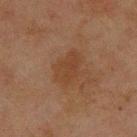Captured during whole-body skin photography for melanoma surveillance; the lesion was not biopsied.
The tile uses cross-polarized illumination.
A close-up tile cropped from a whole-body skin photograph, about 15 mm across.
The lesion's longest dimension is about 4 mm.
Automated image analysis of the tile measured a border-irregularity rating of about 2.5/10, a within-lesion color-variation index near 2.5/10, and radial color variation of about 1.
A male subject, about 45 years old.
From the upper back.